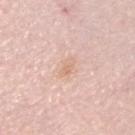biopsy_status: not biopsied; imaged during a skin examination
site: head or neck
lighting: white-light
image:
  source: total-body photography crop
  field_of_view_mm: 15
patient:
  sex: male
  age_approx: 65
automated_metrics:
  area_mm2_approx: 3.0
  eccentricity: 0.9
  shape_asymmetry: 0.25
  cielab_L: 71
  cielab_a: 19
  cielab_b: 30
  vs_skin_darker_L: 6.0
  vs_skin_contrast_norm: 5.0